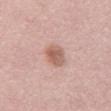| feature | finding |
|---|---|
| workup | imaged on a skin check; not biopsied |
| image source | ~15 mm tile from a whole-body skin photo |
| body site | the mid back |
| patient | female, roughly 65 years of age |
| size | ≈3.5 mm |
| illumination | white-light |
| image-analysis metrics | a lesion color around L≈60 a*≈21 b*≈27 in CIELAB, about 11 CIELAB-L* units darker than the surrounding skin, and a normalized lesion–skin contrast near 7.5; border irregularity of about 1.5 on a 0–10 scale and a color-variation rating of about 3.5/10; a nevus-likeness score of about 75/100 and lesion-presence confidence of about 100/100 |